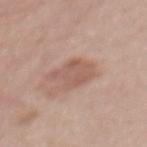Imaged with white-light lighting.
This image is a 15 mm lesion crop taken from a total-body photograph.
Located on the mid back.
The recorded lesion diameter is about 4 mm.
A female subject, about 40 years old.